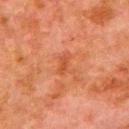Assessment: The lesion was tiled from a total-body skin photograph and was not biopsied. Clinical summary: A lesion tile, about 15 mm wide, cut from a 3D total-body photograph. About 3 mm across. This is a cross-polarized tile. The lesion is located on the left upper arm. Automated image analysis of the tile measured a border-irregularity rating of about 2.5/10, internal color variation of about 0.5 on a 0–10 scale, and peripheral color asymmetry of about 0. And it measured a nevus-likeness score of about 0/100 and a lesion-detection confidence of about 100/100. The patient is a male in their 80s.